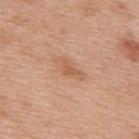Q: Illumination type?
A: white-light illumination
Q: Lesion location?
A: the upper back
Q: How was this image acquired?
A: ~15 mm tile from a whole-body skin photo
Q: Automated lesion metrics?
A: a lesion area of about 2.5 mm², an eccentricity of roughly 0.85, and two-axis asymmetry of about 0.55; a lesion–skin lightness drop of about 8 and a normalized border contrast of about 6; a detector confidence of about 100 out of 100 that the crop contains a lesion
Q: How large is the lesion?
A: ≈2.5 mm
Q: Who is the patient?
A: female, about 40 years old